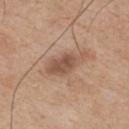{
  "biopsy_status": "not biopsied; imaged during a skin examination",
  "site": "mid back",
  "patient": {
    "sex": "male",
    "age_approx": 75
  },
  "image": {
    "source": "total-body photography crop",
    "field_of_view_mm": 15
  }
}